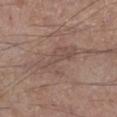  biopsy_status: not biopsied; imaged during a skin examination
  image:
    source: total-body photography crop
    field_of_view_mm: 15
  site: left lower leg
  automated_metrics:
    area_mm2_approx: 9.5
    shape_asymmetry: 0.6
    border_irregularity_0_10: 8.5
    color_variation_0_10: 2.5
    nevus_likeness_0_100: 0
    lesion_detection_confidence_0_100: 70
  lighting: white-light
  patient:
    sex: male
    age_approx: 60
  lesion_size:
    long_diameter_mm_approx: 5.5